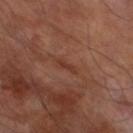anatomic site — the left lower leg; subject — male, in their 70s; tile lighting — cross-polarized illumination; image — ~15 mm crop, total-body skin-cancer survey.